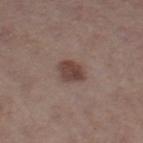Q: Was a biopsy performed?
A: catalogued during a skin exam; not biopsied
Q: What lighting was used for the tile?
A: white-light illumination
Q: Who is the patient?
A: female, in their 40s
Q: What kind of image is this?
A: total-body-photography crop, ~15 mm field of view
Q: How large is the lesion?
A: ~2.5 mm (longest diameter)
Q: Lesion location?
A: the right thigh